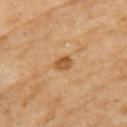Imaged during a routine full-body skin examination; the lesion was not biopsied and no histopathology is available. The subject is a female roughly 70 years of age. Imaged with cross-polarized lighting. A 15 mm crop from a total-body photograph taken for skin-cancer surveillance. The lesion is on the left upper arm. The total-body-photography lesion software estimated an outline eccentricity of about 0.7 (0 = round, 1 = elongated) and a shape-asymmetry score of about 0.25 (0 = symmetric).